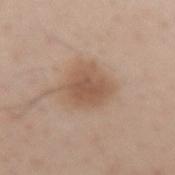<lesion>
<biopsy_status>not biopsied; imaged during a skin examination</biopsy_status>
<patient>
  <sex>female</sex>
  <age_approx>40</age_approx>
</patient>
<site>left forearm</site>
<image>
  <source>total-body photography crop</source>
  <field_of_view_mm>15</field_of_view_mm>
</image>
</lesion>